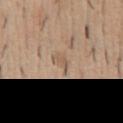Impression: The lesion was photographed on a routine skin check and not biopsied; there is no pathology result. Image and clinical context: A male patient aged 38 to 42. From the abdomen. Measured at roughly 3 mm in maximum diameter. A region of skin cropped from a whole-body photographic capture, roughly 15 mm wide.